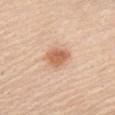Q: Is there a histopathology result?
A: total-body-photography surveillance lesion; no biopsy
Q: Where on the body is the lesion?
A: the front of the torso
Q: What lighting was used for the tile?
A: white-light
Q: What did automated image analysis measure?
A: a mean CIELAB color near L≈63 a*≈23 b*≈34 and a lesion–skin lightness drop of about 12; a border-irregularity index near 1.5/10, internal color variation of about 2.5 on a 0–10 scale, and a peripheral color-asymmetry measure near 1; a detector confidence of about 100 out of 100 that the crop contains a lesion
Q: Who is the patient?
A: male, aged 63 to 67
Q: How was this image acquired?
A: total-body-photography crop, ~15 mm field of view